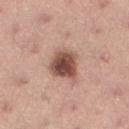Clinical impression:
Imaged during a routine full-body skin examination; the lesion was not biopsied and no histopathology is available.
Clinical summary:
The lesion-visualizer software estimated roughly 18 lightness units darker than nearby skin and a normalized border contrast of about 12. It also reported a border-irregularity index near 2/10, a color-variation rating of about 5.5/10, and peripheral color asymmetry of about 1.5. The subject is a female in their mid- to late 20s. Longest diameter approximately 3.5 mm. On the left lower leg. A 15 mm close-up tile from a total-body photography series done for melanoma screening. The tile uses white-light illumination.